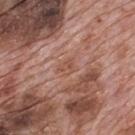Impression:
No biopsy was performed on this lesion — it was imaged during a full skin examination and was not determined to be concerning.
Image and clinical context:
A male subject, aged around 70. A close-up tile cropped from a whole-body skin photograph, about 15 mm across. The lesion is located on the mid back.